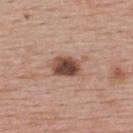The lesion was photographed on a routine skin check and not biopsied; there is no pathology result. From the back. The recorded lesion diameter is about 4 mm. A 15 mm crop from a total-body photograph taken for skin-cancer surveillance. A female subject aged 53–57. The lesion-visualizer software estimated a footprint of about 8 mm² and two-axis asymmetry of about 0.2. The analysis additionally found roughly 16 lightness units darker than nearby skin and a normalized lesion–skin contrast near 11.5. The software also gave a nevus-likeness score of about 95/100.